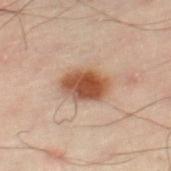| feature | finding |
|---|---|
| acquisition | total-body-photography crop, ~15 mm field of view |
| automated lesion analysis | a border-irregularity index near 1.5/10 and a peripheral color-asymmetry measure near 1.5; an automated nevus-likeness rating near 100 out of 100 and lesion-presence confidence of about 100/100 |
| site | the leg |
| subject | male, approximately 50 years of age |